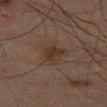<record>
  <biopsy_status>not biopsied; imaged during a skin examination</biopsy_status>
  <patient>
    <sex>male</sex>
    <age_approx>65</age_approx>
  </patient>
  <lesion_size>
    <long_diameter_mm_approx>3.5</long_diameter_mm_approx>
  </lesion_size>
  <automated_metrics>
    <area_mm2_approx>6.0</area_mm2_approx>
    <eccentricity>0.55</eccentricity>
    <shape_asymmetry>0.3</shape_asymmetry>
    <cielab_L>26</cielab_L>
    <cielab_a>13</cielab_a>
    <cielab_b>21</cielab_b>
    <vs_skin_darker_L>6.0</vs_skin_darker_L>
    <vs_skin_contrast_norm>7.0</vs_skin_contrast_norm>
    <border_irregularity_0_10>3.0</border_irregularity_0_10>
    <color_variation_0_10>2.0</color_variation_0_10>
    <peripheral_color_asymmetry>0.5</peripheral_color_asymmetry>
  </automated_metrics>
  <lighting>cross-polarized</lighting>
  <image>
    <source>total-body photography crop</source>
    <field_of_view_mm>15</field_of_view_mm>
  </image>
  <site>left thigh</site>
</record>